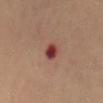Findings:
• workup: imaged on a skin check; not biopsied
• patient: female, aged approximately 60
• image source: 15 mm crop, total-body photography
• anatomic site: the abdomen
• diameter: ~2.5 mm (longest diameter)
• automated metrics: a lesion color around L≈41 a*≈29 b*≈24 in CIELAB; a border-irregularity rating of about 1.5/10, internal color variation of about 5.5 on a 0–10 scale, and radial color variation of about 2
• illumination: cross-polarized